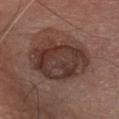This lesion was catalogued during total-body skin photography and was not selected for biopsy. An algorithmic analysis of the crop reported an average lesion color of about L≈36 a*≈18 b*≈22 (CIELAB) and roughly 10 lightness units darker than nearby skin. The software also gave a border-irregularity index near 2/10, internal color variation of about 5.5 on a 0–10 scale, and a peripheral color-asymmetry measure near 2. The software also gave a classifier nevus-likeness of about 15/100. A 15 mm close-up extracted from a 3D total-body photography capture. The lesion's longest dimension is about 8 mm. Imaged with white-light lighting. A male subject aged 73 to 77. On the chest.